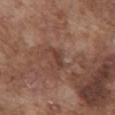biopsy_status: not biopsied; imaged during a skin examination
patient:
  sex: male
  age_approx: 75
lesion_size:
  long_diameter_mm_approx: 3.0
image:
  source: total-body photography crop
  field_of_view_mm: 15
lighting: white-light
automated_metrics:
  color_variation_0_10: 0.0
  peripheral_color_asymmetry: 0.0
  nevus_likeness_0_100: 0
  lesion_detection_confidence_0_100: 85
site: chest